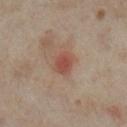biopsy_status: not biopsied; imaged during a skin examination
patient:
  sex: female
  age_approx: 35
site: right lower leg
lesion_size:
  long_diameter_mm_approx: 2.5
image:
  source: total-body photography crop
  field_of_view_mm: 15